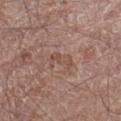{
  "biopsy_status": "not biopsied; imaged during a skin examination",
  "patient": {
    "sex": "male",
    "age_approx": 70
  },
  "site": "left thigh",
  "image": {
    "source": "total-body photography crop",
    "field_of_view_mm": 15
  }
}